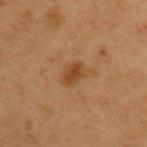| field | value |
|---|---|
| follow-up | total-body-photography surveillance lesion; no biopsy |
| location | the left upper arm |
| patient | female, aged 38–42 |
| lighting | cross-polarized illumination |
| image source | ~15 mm tile from a whole-body skin photo |